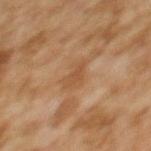| key | value |
|---|---|
| notes | no biopsy performed (imaged during a skin exam) |
| body site | the upper back |
| lesion diameter | ≈3 mm |
| imaging modality | ~15 mm tile from a whole-body skin photo |
| subject | female, aged 58–62 |
| lighting | cross-polarized illumination |